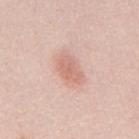Assessment: The lesion was tiled from a total-body skin photograph and was not biopsied. Acquisition and patient details: On the back. The tile uses white-light illumination. Longest diameter approximately 3.5 mm. The subject is a male in their mid- to late 20s. A region of skin cropped from a whole-body photographic capture, roughly 15 mm wide.